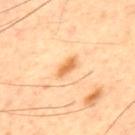Assessment: The lesion was photographed on a routine skin check and not biopsied; there is no pathology result. Acquisition and patient details: The lesion-visualizer software estimated a lesion-to-skin contrast of about 8 (normalized; higher = more distinct). And it measured border irregularity of about 3 on a 0–10 scale, a color-variation rating of about 1.5/10, and peripheral color asymmetry of about 0.5. The recorded lesion diameter is about 3 mm. A male subject, approximately 60 years of age. Imaged with cross-polarized lighting. A 15 mm crop from a total-body photograph taken for skin-cancer surveillance. The lesion is located on the upper back.